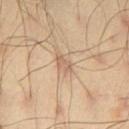Assessment:
This lesion was catalogued during total-body skin photography and was not selected for biopsy.
Background:
The lesion is on the leg. A roughly 15 mm field-of-view crop from a total-body skin photograph. A male patient, about 45 years old.